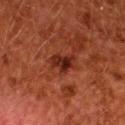Q: Is there a histopathology result?
A: no biopsy performed (imaged during a skin exam)
Q: What are the patient's age and sex?
A: female, aged 48 to 52
Q: What is the lesion's diameter?
A: ≈3 mm
Q: How was the tile lit?
A: cross-polarized
Q: How was this image acquired?
A: 15 mm crop, total-body photography
Q: Lesion location?
A: the upper back